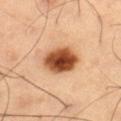Q: Was a biopsy performed?
A: total-body-photography surveillance lesion; no biopsy
Q: Who is the patient?
A: male, approximately 60 years of age
Q: Where on the body is the lesion?
A: the right thigh
Q: What is the imaging modality?
A: ~15 mm tile from a whole-body skin photo
Q: Illumination type?
A: cross-polarized
Q: Lesion size?
A: about 4.5 mm
Q: Automated lesion metrics?
A: a lesion color around L≈50 a*≈26 b*≈38 in CIELAB, roughly 22 lightness units darker than nearby skin, and a normalized lesion–skin contrast near 14; a lesion-detection confidence of about 100/100